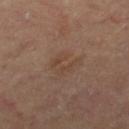biopsy status: catalogued during a skin exam; not biopsied
image-analysis metrics: internal color variation of about 0.5 on a 0–10 scale and radial color variation of about 0.5; an automated nevus-likeness rating near 0 out of 100 and lesion-presence confidence of about 100/100
imaging modality: 15 mm crop, total-body photography
patient: male, in their 60s
location: the left thigh